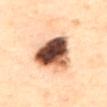A region of skin cropped from a whole-body photographic capture, roughly 15 mm wide.
On the back.
A female patient, aged 58–62.
This is a cross-polarized tile.
The lesion's longest dimension is about 7 mm.